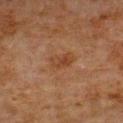The lesion was tiled from a total-body skin photograph and was not biopsied. Located on the left upper arm. The lesion-visualizer software estimated a border-irregularity rating of about 2.5/10, internal color variation of about 2.5 on a 0–10 scale, and a peripheral color-asymmetry measure near 1. It also reported a classifier nevus-likeness of about 5/100 and lesion-presence confidence of about 100/100. The recorded lesion diameter is about 3 mm. A male patient, aged approximately 80. A roughly 15 mm field-of-view crop from a total-body skin photograph. The tile uses cross-polarized illumination.